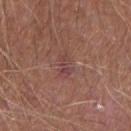No biopsy was performed on this lesion — it was imaged during a full skin examination and was not determined to be concerning.
Automated image analysis of the tile measured two-axis asymmetry of about 0.35. And it measured an automated nevus-likeness rating near 0 out of 100 and a detector confidence of about 100 out of 100 that the crop contains a lesion.
A male patient in their mid-60s.
About 2.5 mm across.
A 15 mm close-up extracted from a 3D total-body photography capture.
Located on the arm.
Imaged with white-light lighting.